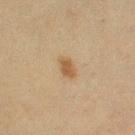Image and clinical context: A female patient, aged around 55. A close-up tile cropped from a whole-body skin photograph, about 15 mm across. The lesion is located on the leg.